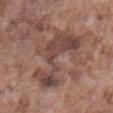The lesion was photographed on a routine skin check and not biopsied; there is no pathology result.
This image is a 15 mm lesion crop taken from a total-body photograph.
A male subject aged 73 to 77.
From the abdomen.